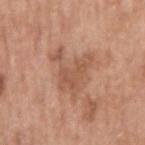  patient:
    sex: male
    age_approx: 65
  image:
    source: total-body photography crop
    field_of_view_mm: 15
  lighting: white-light
  site: arm
  automated_metrics:
    cielab_L: 55
    cielab_a: 22
    cielab_b: 31
    vs_skin_darker_L: 8.0
    vs_skin_contrast_norm: 6.0
    peripheral_color_asymmetry: 0.5
    nevus_likeness_0_100: 0